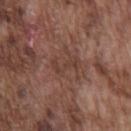  biopsy_status: not biopsied; imaged during a skin examination
  lesion_size:
    long_diameter_mm_approx: 3.5
  automated_metrics:
    border_irregularity_0_10: 6.0
    color_variation_0_10: 1.5
    peripheral_color_asymmetry: 0.5
    nevus_likeness_0_100: 0
  image:
    source: total-body photography crop
    field_of_view_mm: 15
  lighting: white-light
  site: chest
  patient:
    sex: male
    age_approx: 75